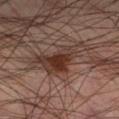Impression:
This lesion was catalogued during total-body skin photography and was not selected for biopsy.
Context:
Cropped from a total-body skin-imaging series; the visible field is about 15 mm. The lesion is on the right lower leg. A male subject aged approximately 45. The recorded lesion diameter is about 7.5 mm. Imaged with cross-polarized lighting. The total-body-photography lesion software estimated a footprint of about 15 mm² and a shape-asymmetry score of about 0.45 (0 = symmetric). The analysis additionally found a normalized lesion–skin contrast near 9. And it measured border irregularity of about 6.5 on a 0–10 scale, internal color variation of about 5.5 on a 0–10 scale, and peripheral color asymmetry of about 1.5. The analysis additionally found a classifier nevus-likeness of about 75/100.